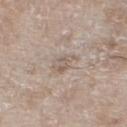Part of a total-body skin-imaging series; this lesion was reviewed on a skin check and was not flagged for biopsy. The lesion is located on the left lower leg. A female patient, aged around 75. A 15 mm close-up extracted from a 3D total-body photography capture.